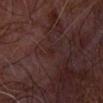Findings:
– biopsy status: imaged on a skin check; not biopsied
– image source: ~15 mm tile from a whole-body skin photo
– patient: male, about 65 years old
– lesion size: ~1.5 mm (longest diameter)
– site: the left forearm